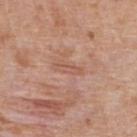The tile uses white-light illumination.
The patient is a female about 40 years old.
Measured at roughly 3.5 mm in maximum diameter.
A region of skin cropped from a whole-body photographic capture, roughly 15 mm wide.
From the upper back.